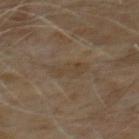No biopsy was performed on this lesion — it was imaged during a full skin examination and was not determined to be concerning. Measured at roughly 4 mm in maximum diameter. The lesion is located on the chest. The total-body-photography lesion software estimated a color-variation rating of about 1.5/10 and radial color variation of about 0.5. The software also gave an automated nevus-likeness rating near 0 out of 100 and a lesion-detection confidence of about 90/100. Captured under cross-polarized illumination. A male subject roughly 60 years of age. Cropped from a total-body skin-imaging series; the visible field is about 15 mm.